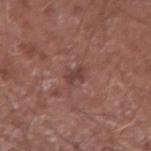Clinical summary:
Cropped from a whole-body photographic skin survey; the tile spans about 15 mm. Located on the arm. A male subject, approximately 65 years of age. The lesion-visualizer software estimated an area of roughly 3 mm², an eccentricity of roughly 0.85, and a symmetry-axis asymmetry near 0.4. It also reported a mean CIELAB color near L≈41 a*≈22 b*≈22, roughly 8 lightness units darker than nearby skin, and a lesion-to-skin contrast of about 6.5 (normalized; higher = more distinct). The software also gave border irregularity of about 4 on a 0–10 scale, internal color variation of about 0 on a 0–10 scale, and a peripheral color-asymmetry measure near 0. The recorded lesion diameter is about 2.5 mm. Captured under white-light illumination.